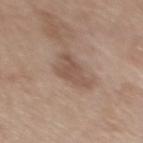The lesion was photographed on a routine skin check and not biopsied; there is no pathology result.